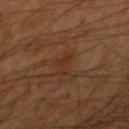Clinical summary: On the right upper arm. A male subject, approximately 35 years of age. Cropped from a whole-body photographic skin survey; the tile spans about 15 mm.